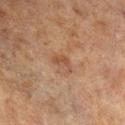Impression: Recorded during total-body skin imaging; not selected for excision or biopsy. Acquisition and patient details: A 15 mm close-up tile from a total-body photography series done for melanoma screening. Approximately 3 mm at its widest. The patient is a male aged around 70. On the right lower leg. The tile uses cross-polarized illumination. The total-body-photography lesion software estimated a border-irregularity index near 5/10, a color-variation rating of about 1/10, and peripheral color asymmetry of about 0.5.